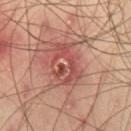| feature | finding |
|---|---|
| follow-up | catalogued during a skin exam; not biopsied |
| lesion size | ≈5 mm |
| image source | ~15 mm tile from a whole-body skin photo |
| lighting | cross-polarized illumination |
| TBP lesion metrics | an area of roughly 13 mm², an eccentricity of roughly 0.7, and a shape-asymmetry score of about 0.25 (0 = symmetric); border irregularity of about 3 on a 0–10 scale, a color-variation rating of about 9.5/10, and radial color variation of about 3.5; a classifier nevus-likeness of about 0/100 |
| anatomic site | the right thigh |
| patient | male, aged 38–42 |